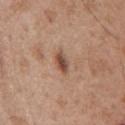The lesion was tiled from a total-body skin photograph and was not biopsied. A male patient, in their mid- to late 50s. About 2.5 mm across. On the chest. A 15 mm close-up extracted from a 3D total-body photography capture.